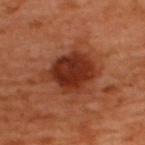<tbp_lesion>
<biopsy_status>not biopsied; imaged during a skin examination</biopsy_status>
<patient>
  <sex>male</sex>
  <age_approx>50</age_approx>
</patient>
<site>back</site>
<lesion_size>
  <long_diameter_mm_approx>6.0</long_diameter_mm_approx>
</lesion_size>
<lighting>cross-polarized</lighting>
<image>
  <source>total-body photography crop</source>
  <field_of_view_mm>15</field_of_view_mm>
</image>
</tbp_lesion>